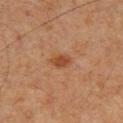notes: imaged on a skin check; not biopsied | image: ~15 mm tile from a whole-body skin photo | illumination: cross-polarized illumination | patient: male, roughly 60 years of age | site: the upper back | size: ≈2.5 mm.